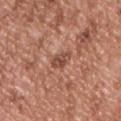{"biopsy_status": "not biopsied; imaged during a skin examination", "lesion_size": {"long_diameter_mm_approx": 3.0}, "automated_metrics": {"color_variation_0_10": 3.0, "peripheral_color_asymmetry": 1.0, "nevus_likeness_0_100": 0, "lesion_detection_confidence_0_100": 100}, "lighting": "white-light", "site": "chest", "patient": {"sex": "male", "age_approx": 55}, "image": {"source": "total-body photography crop", "field_of_view_mm": 15}}